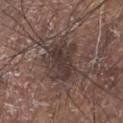Recorded during total-body skin imaging; not selected for excision or biopsy.
A male patient, in their 80s.
A roughly 15 mm field-of-view crop from a total-body skin photograph.
The lesion is located on the chest.
The lesion's longest dimension is about 5 mm.
This is a white-light tile.